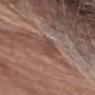Notes:
– follow-up: total-body-photography surveillance lesion; no biopsy
– location: the abdomen
– tile lighting: white-light illumination
– image source: total-body-photography crop, ~15 mm field of view
– patient: female, aged around 65
– lesion size: ≈3 mm